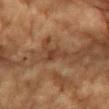{
  "biopsy_status": "not biopsied; imaged during a skin examination",
  "automated_metrics": {
    "vs_skin_darker_L": 7.0,
    "vs_skin_contrast_norm": 6.5,
    "color_variation_0_10": 1.0,
    "peripheral_color_asymmetry": 0.0
  },
  "lesion_size": {
    "long_diameter_mm_approx": 3.0
  },
  "site": "chest",
  "image": {
    "source": "total-body photography crop",
    "field_of_view_mm": 15
  },
  "lighting": "cross-polarized",
  "patient": {
    "sex": "male",
    "age_approx": "85+"
  }
}